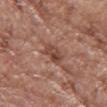Q: Is there a histopathology result?
A: imaged on a skin check; not biopsied
Q: What kind of image is this?
A: ~15 mm crop, total-body skin-cancer survey
Q: Illumination type?
A: white-light illumination
Q: What is the lesion's diameter?
A: about 3.5 mm
Q: What are the patient's age and sex?
A: female, aged approximately 65
Q: Where on the body is the lesion?
A: the chest
Q: What did automated image analysis measure?
A: a shape eccentricity near 0.95 and a shape-asymmetry score of about 0.25 (0 = symmetric); a normalized border contrast of about 8; internal color variation of about 4.5 on a 0–10 scale and radial color variation of about 2; a nevus-likeness score of about 0/100 and lesion-presence confidence of about 75/100